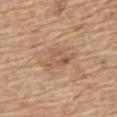biopsy status = imaged on a skin check; not biopsied
imaging modality = total-body-photography crop, ~15 mm field of view
tile lighting = white-light
diameter = ≈4.5 mm
subject = male, in their 70s
anatomic site = the chest